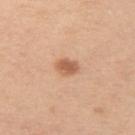| key | value |
|---|---|
| lighting | white-light |
| image source | ~15 mm crop, total-body skin-cancer survey |
| subject | female, approximately 40 years of age |
| lesion size | ~2.5 mm (longest diameter) |
| anatomic site | the left upper arm |
| TBP lesion metrics | a lesion area of about 4.5 mm²; a lesion color around L≈60 a*≈23 b*≈34 in CIELAB and about 12 CIELAB-L* units darker than the surrounding skin; internal color variation of about 2.5 on a 0–10 scale and peripheral color asymmetry of about 1; an automated nevus-likeness rating near 95 out of 100 and a detector confidence of about 100 out of 100 that the crop contains a lesion |